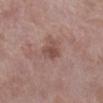Acquisition and patient details: The lesion is located on the right lower leg. A close-up tile cropped from a whole-body skin photograph, about 15 mm across. The patient is a female aged approximately 70. Imaged with white-light lighting. The lesion's longest dimension is about 3 mm. Automated tile analysis of the lesion measured an eccentricity of roughly 0.65 and a shape-asymmetry score of about 0.4 (0 = symmetric). And it measured a lesion color around L≈48 a*≈20 b*≈24 in CIELAB and roughly 9 lightness units darker than nearby skin. The analysis additionally found a classifier nevus-likeness of about 0/100 and a lesion-detection confidence of about 100/100.